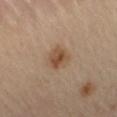biopsy status: total-body-photography surveillance lesion; no biopsy | image source: 15 mm crop, total-body photography | lesion size: ≈3.5 mm | anatomic site: the right thigh | automated lesion analysis: a lesion area of about 6.5 mm², an outline eccentricity of about 0.65 (0 = round, 1 = elongated), and a shape-asymmetry score of about 0.25 (0 = symmetric); a nevus-likeness score of about 90/100 and a lesion-detection confidence of about 100/100 | patient: male, aged 63 to 67 | tile lighting: cross-polarized.